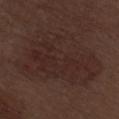tile lighting: white-light
image: ~15 mm crop, total-body skin-cancer survey
site: the right thigh
size: about 11 mm
automated metrics: a classifier nevus-likeness of about 10/100 and lesion-presence confidence of about 100/100
subject: male, aged 68 to 72